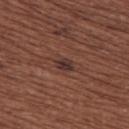Clinical impression: Part of a total-body skin-imaging series; this lesion was reviewed on a skin check and was not flagged for biopsy. Acquisition and patient details: Located on the back. A 15 mm crop from a total-body photograph taken for skin-cancer surveillance. Longest diameter approximately 3 mm. The total-body-photography lesion software estimated a footprint of about 4 mm² and a symmetry-axis asymmetry near 0.2. The analysis additionally found border irregularity of about 2 on a 0–10 scale, internal color variation of about 2.5 on a 0–10 scale, and a peripheral color-asymmetry measure near 1. And it measured a nevus-likeness score of about 5/100 and a lesion-detection confidence of about 100/100. The tile uses white-light illumination. A female subject aged 63 to 67.